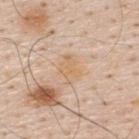workup: no biopsy performed (imaged during a skin exam)
size: about 3.5 mm
tile lighting: white-light
TBP lesion metrics: an area of roughly 6 mm², an outline eccentricity of about 0.7 (0 = round, 1 = elongated), and a shape-asymmetry score of about 0.25 (0 = symmetric)
acquisition: ~15 mm crop, total-body skin-cancer survey
patient: male, aged 58 to 62
anatomic site: the upper back